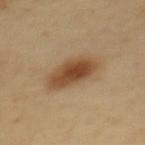Clinical impression:
Imaged during a routine full-body skin examination; the lesion was not biopsied and no histopathology is available.
Image and clinical context:
The lesion-visualizer software estimated a footprint of about 12 mm², a shape eccentricity near 0.8, and a shape-asymmetry score of about 0.2 (0 = symmetric). The software also gave a detector confidence of about 100 out of 100 that the crop contains a lesion. Measured at roughly 5.5 mm in maximum diameter. From the back. The subject is a female about 60 years old. A 15 mm crop from a total-body photograph taken for skin-cancer surveillance.